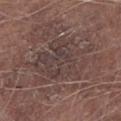• notes: catalogued during a skin exam; not biopsied
• lighting: white-light illumination
• body site: the left lower leg
• subject: male, aged 73 to 77
• image: ~15 mm crop, total-body skin-cancer survey